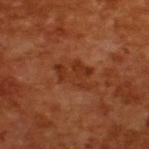notes: catalogued during a skin exam; not biopsied
subject: male, aged 63 to 67
acquisition: ~15 mm crop, total-body skin-cancer survey
automated metrics: an average lesion color of about L≈33 a*≈26 b*≈33 (CIELAB), about 7 CIELAB-L* units darker than the surrounding skin, and a lesion-to-skin contrast of about 6.5 (normalized; higher = more distinct)
lighting: cross-polarized